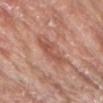This lesion was catalogued during total-body skin photography and was not selected for biopsy. An algorithmic analysis of the crop reported a border-irregularity rating of about 4.5/10 and radial color variation of about 1. The software also gave a classifier nevus-likeness of about 0/100. Cropped from a total-body skin-imaging series; the visible field is about 15 mm. A male subject, aged approximately 65. The tile uses white-light illumination. On the chest. Longest diameter approximately 6 mm.